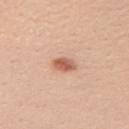biopsy_status: not biopsied; imaged during a skin examination
lesion_size:
  long_diameter_mm_approx: 2.5
patient:
  sex: female
  age_approx: 25
image:
  source: total-body photography crop
  field_of_view_mm: 15
automated_metrics:
  cielab_L: 60
  cielab_a: 25
  cielab_b: 32
  vs_skin_darker_L: 13.0
  nevus_likeness_0_100: 95
  lesion_detection_confidence_0_100: 100
lighting: white-light
site: left upper arm